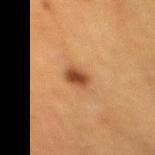This lesion was catalogued during total-body skin photography and was not selected for biopsy.
Automated tile analysis of the lesion measured a footprint of about 4.5 mm² and a symmetry-axis asymmetry near 0.15. It also reported a mean CIELAB color near L≈39 a*≈21 b*≈32, roughly 12 lightness units darker than nearby skin, and a normalized lesion–skin contrast near 10. The analysis additionally found a classifier nevus-likeness of about 100/100 and a detector confidence of about 100 out of 100 that the crop contains a lesion.
A female patient about 40 years old.
Captured under cross-polarized illumination.
A 15 mm crop from a total-body photograph taken for skin-cancer surveillance.
Located on the leg.